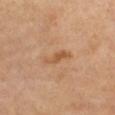Case summary:
- notes: catalogued during a skin exam; not biopsied
- patient: female, in their 60s
- image source: total-body-photography crop, ~15 mm field of view
- anatomic site: the chest
- size: ~4 mm (longest diameter)
- automated metrics: an eccentricity of roughly 0.95 and two-axis asymmetry of about 0.35; a lesion color around L≈55 a*≈21 b*≈37 in CIELAB and a lesion-to-skin contrast of about 6.5 (normalized; higher = more distinct)
- lighting: cross-polarized